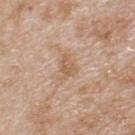Findings:
• subject — male, roughly 80 years of age
• automated metrics — a mean CIELAB color near L≈59 a*≈18 b*≈32, roughly 8 lightness units darker than nearby skin, and a normalized border contrast of about 6; a border-irregularity rating of about 3.5/10, a color-variation rating of about 2.5/10, and peripheral color asymmetry of about 1; lesion-presence confidence of about 100/100
• location — the upper back
• acquisition — ~15 mm tile from a whole-body skin photo